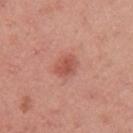Q: Was this lesion biopsied?
A: imaged on a skin check; not biopsied
Q: Automated lesion metrics?
A: a footprint of about 5 mm², an eccentricity of roughly 0.75, and a shape-asymmetry score of about 0.2 (0 = symmetric); an average lesion color of about L≈54 a*≈28 b*≈30 (CIELAB) and about 9 CIELAB-L* units darker than the surrounding skin
Q: Lesion location?
A: the left upper arm
Q: Patient demographics?
A: female, about 50 years old
Q: What is the imaging modality?
A: total-body-photography crop, ~15 mm field of view
Q: Lesion size?
A: ≈3 mm